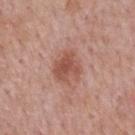Part of a total-body skin-imaging series; this lesion was reviewed on a skin check and was not flagged for biopsy.
This image is a 15 mm lesion crop taken from a total-body photograph.
From the back.
The subject is a male in their mid- to late 50s.